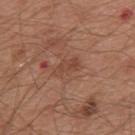Q: Was this lesion biopsied?
A: total-body-photography surveillance lesion; no biopsy
Q: What kind of image is this?
A: 15 mm crop, total-body photography
Q: Lesion location?
A: the mid back
Q: Patient demographics?
A: male, in their mid- to late 60s